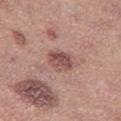biopsy status=imaged on a skin check; not biopsied | patient=female, roughly 40 years of age | size=~3 mm (longest diameter) | location=the left thigh | automated metrics=border irregularity of about 2 on a 0–10 scale, a color-variation rating of about 5/10, and radial color variation of about 1.5 | tile lighting=white-light | image=total-body-photography crop, ~15 mm field of view.